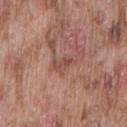– follow-up — total-body-photography surveillance lesion; no biopsy
– lesion size — ~3 mm (longest diameter)
– imaging modality — 15 mm crop, total-body photography
– body site — the lower back
– subject — male, roughly 75 years of age
– automated lesion analysis — a lesion area of about 4 mm², an eccentricity of roughly 0.85, and a shape-asymmetry score of about 0.35 (0 = symmetric); a border-irregularity rating of about 4/10, a color-variation rating of about 3.5/10, and a peripheral color-asymmetry measure near 1; a classifier nevus-likeness of about 0/100 and a lesion-detection confidence of about 90/100